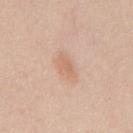| key | value |
|---|---|
| workup | no biopsy performed (imaged during a skin exam) |
| imaging modality | ~15 mm crop, total-body skin-cancer survey |
| site | the mid back |
| lighting | white-light |
| patient | male, approximately 60 years of age |
| diameter | ~3 mm (longest diameter) |